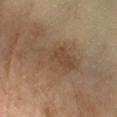notes=no biopsy performed (imaged during a skin exam) | image source=15 mm crop, total-body photography | size=about 6 mm | patient=female, about 70 years old | automated metrics=an area of roughly 15 mm² and a shape eccentricity near 0.8 | site=the right forearm | illumination=cross-polarized.